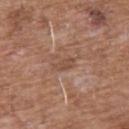| key | value |
|---|---|
| notes | no biopsy performed (imaged during a skin exam) |
| patient | male, roughly 60 years of age |
| image | 15 mm crop, total-body photography |
| site | the front of the torso |